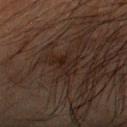<record>
<biopsy_status>not biopsied; imaged during a skin examination</biopsy_status>
<site>left forearm</site>
<lighting>cross-polarized</lighting>
<automated_metrics>
  <area_mm2_approx>4.5</area_mm2_approx>
  <shape_asymmetry>0.4</shape_asymmetry>
  <color_variation_0_10>3.0</color_variation_0_10>
  <peripheral_color_asymmetry>1.0</peripheral_color_asymmetry>
  <nevus_likeness_0_100>0</nevus_likeness_0_100>
  <lesion_detection_confidence_0_100>95</lesion_detection_confidence_0_100>
</automated_metrics>
<patient>
  <sex>male</sex>
  <age_approx>65</age_approx>
</patient>
<lesion_size>
  <long_diameter_mm_approx>2.5</long_diameter_mm_approx>
</lesion_size>
<image>
  <source>total-body photography crop</source>
  <field_of_view_mm>15</field_of_view_mm>
</image>
</record>